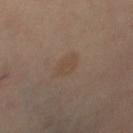Clinical impression: Captured during whole-body skin photography for melanoma surveillance; the lesion was not biopsied. Background: Located on the right thigh. A close-up tile cropped from a whole-body skin photograph, about 15 mm across. The subject is a female about 50 years old.